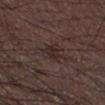workup = no biopsy performed (imaged during a skin exam) | patient = male, approximately 50 years of age | image source = 15 mm crop, total-body photography | size = about 2.5 mm | TBP lesion metrics = a shape eccentricity near 0.7 and two-axis asymmetry of about 0.35; a lesion–skin lightness drop of about 6 and a normalized lesion–skin contrast near 7; border irregularity of about 3 on a 0–10 scale, a within-lesion color-variation index near 1.5/10, and radial color variation of about 0.5; a nevus-likeness score of about 0/100 and a lesion-detection confidence of about 100/100 | site = the left lower leg | illumination = white-light.